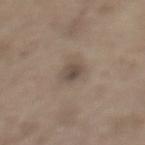Findings:
• workup: total-body-photography surveillance lesion; no biopsy
• acquisition: ~15 mm crop, total-body skin-cancer survey
• anatomic site: the left lower leg
• subject: male, aged 68–72
• TBP lesion metrics: an automated nevus-likeness rating near 30 out of 100 and a lesion-detection confidence of about 100/100